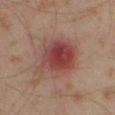A male patient approximately 60 years of age. Automated image analysis of the tile measured a lesion area of about 21 mm², a shape eccentricity near 0.5, and a shape-asymmetry score of about 0.2 (0 = symmetric). It also reported a classifier nevus-likeness of about 0/100 and a lesion-detection confidence of about 100/100. Longest diameter approximately 5.5 mm. This is a cross-polarized tile. On the leg. A 15 mm crop from a total-body photograph taken for skin-cancer surveillance.